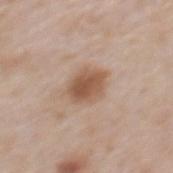An algorithmic analysis of the crop reported a lesion color around L≈54 a*≈19 b*≈30 in CIELAB, a lesion–skin lightness drop of about 11, and a normalized lesion–skin contrast near 8.5. On the mid back. A 15 mm close-up extracted from a 3D total-body photography capture. A male subject, roughly 65 years of age. The tile uses white-light illumination. Longest diameter approximately 4 mm.